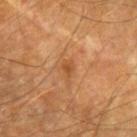Notes:
• notes — no biopsy performed (imaged during a skin exam)
• anatomic site — the right forearm
• image — 15 mm crop, total-body photography
• tile lighting — cross-polarized
• lesion size — ≈1.5 mm
• automated metrics — a lesion area of about 1.5 mm² and an outline eccentricity of about 0.55 (0 = round, 1 = elongated); a nevus-likeness score of about 0/100 and a lesion-detection confidence of about 100/100
• patient — male, approximately 65 years of age